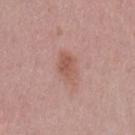Impression: Imaged during a routine full-body skin examination; the lesion was not biopsied and no histopathology is available. Background: A female patient, roughly 40 years of age. This is a white-light tile. The lesion is on the left thigh. A region of skin cropped from a whole-body photographic capture, roughly 15 mm wide. Automated image analysis of the tile measured a lesion area of about 6 mm², an outline eccentricity of about 0.75 (0 = round, 1 = elongated), and a symmetry-axis asymmetry near 0.2. The analysis additionally found a lesion color around L≈55 a*≈22 b*≈27 in CIELAB, a lesion–skin lightness drop of about 8, and a lesion-to-skin contrast of about 6.5 (normalized; higher = more distinct). The software also gave a classifier nevus-likeness of about 40/100 and a detector confidence of about 100 out of 100 that the crop contains a lesion.